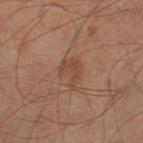follow-up: total-body-photography surveillance lesion; no biopsy
location: the leg
patient: male, roughly 60 years of age
automated lesion analysis: a footprint of about 6 mm², an eccentricity of roughly 0.75, and a shape-asymmetry score of about 0.45 (0 = symmetric); a mean CIELAB color near L≈40 a*≈18 b*≈26, a lesion–skin lightness drop of about 6, and a normalized lesion–skin contrast near 5.5; an automated nevus-likeness rating near 15 out of 100 and a detector confidence of about 100 out of 100 that the crop contains a lesion
image: ~15 mm crop, total-body skin-cancer survey
tile lighting: cross-polarized illumination